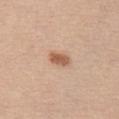The lesion was tiled from a total-body skin photograph and was not biopsied. Automated image analysis of the tile measured a lesion area of about 4 mm², an eccentricity of roughly 0.85, and a shape-asymmetry score of about 0.25 (0 = symmetric). The analysis additionally found a border-irregularity index near 2.5/10, a within-lesion color-variation index near 2/10, and a peripheral color-asymmetry measure near 0.5. Longest diameter approximately 3 mm. Located on the front of the torso. A 15 mm close-up extracted from a 3D total-body photography capture. A male patient roughly 30 years of age.